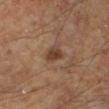Findings:
- notes · catalogued during a skin exam; not biopsied
- patient · male, about 60 years old
- image · total-body-photography crop, ~15 mm field of view
- lighting · cross-polarized
- size · about 3 mm
- site · the leg
- automated metrics · a mean CIELAB color near L≈37 a*≈17 b*≈25 and a normalized border contrast of about 7.5; a within-lesion color-variation index near 2.5/10; a nevus-likeness score of about 80/100